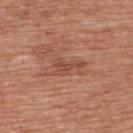Part of a total-body skin-imaging series; this lesion was reviewed on a skin check and was not flagged for biopsy. Automated image analysis of the tile measured an area of roughly 4.5 mm² and two-axis asymmetry of about 0.65. And it measured a mean CIELAB color near L≈48 a*≈25 b*≈29, roughly 8 lightness units darker than nearby skin, and a lesion-to-skin contrast of about 6 (normalized; higher = more distinct). A region of skin cropped from a whole-body photographic capture, roughly 15 mm wide. A male subject in their 70s. The lesion is on the back.